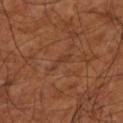Recorded during total-body skin imaging; not selected for excision or biopsy. The patient is roughly 65 years of age. Located on the left lower leg. Automated tile analysis of the lesion measured a footprint of about 2 mm² and an eccentricity of roughly 0.95. The lesion's longest dimension is about 3 mm. Cropped from a whole-body photographic skin survey; the tile spans about 15 mm.